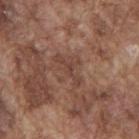Assessment: This lesion was catalogued during total-body skin photography and was not selected for biopsy. Acquisition and patient details: The lesion is on the mid back. Automated image analysis of the tile measured a footprint of about 4.5 mm², an outline eccentricity of about 0.9 (0 = round, 1 = elongated), and two-axis asymmetry of about 0.55. The software also gave a mean CIELAB color near L≈42 a*≈20 b*≈25, about 7 CIELAB-L* units darker than the surrounding skin, and a lesion-to-skin contrast of about 5.5 (normalized; higher = more distinct). Captured under white-light illumination. The lesion's longest dimension is about 4.5 mm. A male subject about 75 years old. A 15 mm close-up tile from a total-body photography series done for melanoma screening.